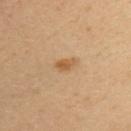notes: total-body-photography surveillance lesion; no biopsy.